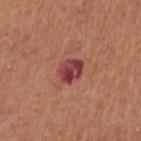Q: Is there a histopathology result?
A: catalogued during a skin exam; not biopsied
Q: What is the imaging modality?
A: 15 mm crop, total-body photography
Q: Lesion location?
A: the arm
Q: How large is the lesion?
A: ~3 mm (longest diameter)
Q: What are the patient's age and sex?
A: female, approximately 65 years of age
Q: How was the tile lit?
A: white-light